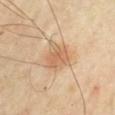Clinical impression:
Recorded during total-body skin imaging; not selected for excision or biopsy.
Context:
Imaged with cross-polarized lighting. A 15 mm close-up extracted from a 3D total-body photography capture. On the right upper arm. A male subject in their mid- to late 60s. Measured at roughly 4 mm in maximum diameter.